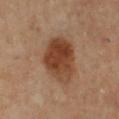follow-up = no biopsy performed (imaged during a skin exam)
tile lighting = cross-polarized illumination
site = the left leg
automated lesion analysis = an average lesion color of about L≈36 a*≈19 b*≈28 (CIELAB), roughly 11 lightness units darker than nearby skin, and a normalized border contrast of about 10; a border-irregularity index near 2/10, a color-variation rating of about 5/10, and radial color variation of about 1.5; an automated nevus-likeness rating near 95 out of 100
imaging modality = total-body-photography crop, ~15 mm field of view
patient = female, aged 58–62
size = about 5 mm